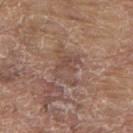biopsy_status: not biopsied; imaged during a skin examination